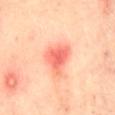Assessment: Captured during whole-body skin photography for melanoma surveillance; the lesion was not biopsied. Context: Located on the back. A close-up tile cropped from a whole-body skin photograph, about 15 mm across. A female subject aged 38 to 42.